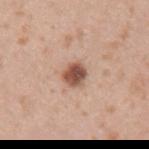Clinical impression: This lesion was catalogued during total-body skin photography and was not selected for biopsy. Clinical summary: From the left upper arm. The subject is a male in their mid-30s. Imaged with white-light lighting. Approximately 2.5 mm at its widest. A roughly 15 mm field-of-view crop from a total-body skin photograph. Automated image analysis of the tile measured a border-irregularity index near 2.5/10, internal color variation of about 5 on a 0–10 scale, and a peripheral color-asymmetry measure near 1.5. And it measured an automated nevus-likeness rating near 95 out of 100 and a lesion-detection confidence of about 100/100.